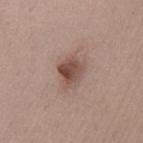Q: Was a biopsy performed?
A: total-body-photography surveillance lesion; no biopsy
Q: Lesion size?
A: ~3.5 mm (longest diameter)
Q: What are the patient's age and sex?
A: male, about 40 years old
Q: Lesion location?
A: the back
Q: What lighting was used for the tile?
A: white-light
Q: How was this image acquired?
A: ~15 mm crop, total-body skin-cancer survey